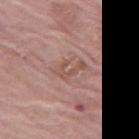{
  "biopsy_status": "not biopsied; imaged during a skin examination",
  "image": {
    "source": "total-body photography crop",
    "field_of_view_mm": 15
  },
  "lesion_size": {
    "long_diameter_mm_approx": 3.5
  },
  "site": "left thigh",
  "lighting": "white-light",
  "automated_metrics": {
    "area_mm2_approx": 5.0,
    "cielab_L": 53,
    "cielab_a": 20,
    "cielab_b": 24,
    "border_irregularity_0_10": 7.5,
    "color_variation_0_10": 0.0,
    "peripheral_color_asymmetry": 0.0
  },
  "patient": {
    "sex": "female",
    "age_approx": "85+"
  }
}